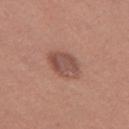Assessment:
The lesion was tiled from a total-body skin photograph and was not biopsied.
Context:
The total-body-photography lesion software estimated a footprint of about 9 mm², an outline eccentricity of about 0.75 (0 = round, 1 = elongated), and a shape-asymmetry score of about 0.1 (0 = symmetric). And it measured a nevus-likeness score of about 45/100. A lesion tile, about 15 mm wide, cut from a 3D total-body photograph. A female patient roughly 30 years of age. The lesion's longest dimension is about 4 mm. From the left thigh.